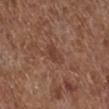Case summary:
* notes: no biopsy performed (imaged during a skin exam)
* subject: male, aged 73–77
* diameter: about 2.5 mm
* tile lighting: white-light
* body site: the right lower leg
* acquisition: ~15 mm tile from a whole-body skin photo
* TBP lesion metrics: a footprint of about 3.5 mm², an outline eccentricity of about 0.8 (0 = round, 1 = elongated), and a shape-asymmetry score of about 0.2 (0 = symmetric); a border-irregularity rating of about 1.5/10, a color-variation rating of about 2/10, and peripheral color asymmetry of about 0.5